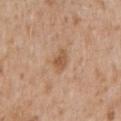biopsy_status: not biopsied; imaged during a skin examination
site: front of the torso
image:
  source: total-body photography crop
  field_of_view_mm: 15
lighting: white-light
patient:
  sex: male
  age_approx: 65